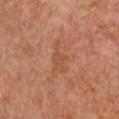{
  "biopsy_status": "not biopsied; imaged during a skin examination",
  "patient": {
    "sex": "female",
    "age_approx": 55
  },
  "image": {
    "source": "total-body photography crop",
    "field_of_view_mm": 15
  },
  "automated_metrics": {
    "border_irregularity_0_10": 5.5,
    "color_variation_0_10": 1.0,
    "nevus_likeness_0_100": 0
  },
  "site": "chest"
}